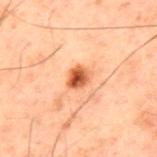Recorded during total-body skin imaging; not selected for excision or biopsy. A male patient about 60 years old. A close-up tile cropped from a whole-body skin photograph, about 15 mm across. The tile uses cross-polarized illumination. From the upper back.